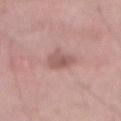Q: Was a biopsy performed?
A: no biopsy performed (imaged during a skin exam)
Q: What lighting was used for the tile?
A: white-light illumination
Q: What did automated image analysis measure?
A: a lesion area of about 5.5 mm², an eccentricity of roughly 0.65, and a shape-asymmetry score of about 0.25 (0 = symmetric); a lesion color around L≈55 a*≈21 b*≈22 in CIELAB, a lesion–skin lightness drop of about 9, and a normalized lesion–skin contrast near 6.5; a border-irregularity rating of about 2.5/10 and a within-lesion color-variation index near 3/10; an automated nevus-likeness rating near 0 out of 100 and a lesion-detection confidence of about 100/100
Q: What is the anatomic site?
A: the abdomen
Q: What are the patient's age and sex?
A: male, approximately 50 years of age
Q: Lesion size?
A: about 3.5 mm
Q: What is the imaging modality?
A: ~15 mm crop, total-body skin-cancer survey